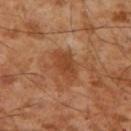A 15 mm close-up tile from a total-body photography series done for melanoma screening. This is a cross-polarized tile. The patient is a male in their mid-50s. Approximately 4.5 mm at its widest. The lesion is on the right upper arm.